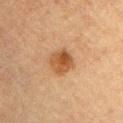The lesion was photographed on a routine skin check and not biopsied; there is no pathology result. The patient is a female about 55 years old. Imaged with cross-polarized lighting. This image is a 15 mm lesion crop taken from a total-body photograph. The lesion is on the chest.